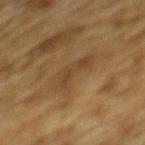follow-up = catalogued during a skin exam; not biopsied | lighting = cross-polarized illumination | subject = male, approximately 85 years of age or older | image-analysis metrics = a within-lesion color-variation index near 2.5/10; a classifier nevus-likeness of about 0/100 and a lesion-detection confidence of about 65/100 | lesion diameter = ~4 mm (longest diameter) | image = 15 mm crop, total-body photography | anatomic site = the upper back.